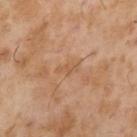Case summary:
• workup: no biopsy performed (imaged during a skin exam)
• image source: ~15 mm tile from a whole-body skin photo
• lighting: cross-polarized illumination
• anatomic site: the arm
• lesion size: about 3 mm
• automated lesion analysis: a border-irregularity index near 4/10 and a color-variation rating of about 0.5/10; a detector confidence of about 100 out of 100 that the crop contains a lesion
• patient: male, roughly 55 years of age